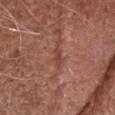biopsy_status: not biopsied; imaged during a skin examination
automated_metrics:
  eccentricity: 0.95
  shape_asymmetry: 0.35
lighting: white-light
lesion_size:
  long_diameter_mm_approx: 3.0
patient:
  sex: male
  age_approx: 75
site: head or neck
image:
  source: total-body photography crop
  field_of_view_mm: 15